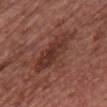Findings:
* follow-up — catalogued during a skin exam; not biopsied
* lesion diameter — ~7 mm (longest diameter)
* lighting — white-light
* anatomic site — the chest
* image — 15 mm crop, total-body photography
* automated lesion analysis — a border-irregularity index near 5/10, internal color variation of about 5 on a 0–10 scale, and radial color variation of about 1.5; a nevus-likeness score of about 20/100
* subject — female, roughly 65 years of age